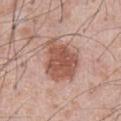biopsy_status: not biopsied; imaged during a skin examination
patient:
  sex: male
  age_approx: 55
site: abdomen
image:
  source: total-body photography crop
  field_of_view_mm: 15